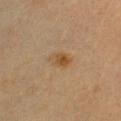workup: imaged on a skin check; not biopsied
acquisition: ~15 mm crop, total-body skin-cancer survey
tile lighting: cross-polarized illumination
patient: female, about 20 years old
image-analysis metrics: a footprint of about 5 mm², an outline eccentricity of about 0.75 (0 = round, 1 = elongated), and a symmetry-axis asymmetry near 0.1; a lesion color around L≈44 a*≈16 b*≈32 in CIELAB, about 7 CIELAB-L* units darker than the surrounding skin, and a lesion-to-skin contrast of about 7 (normalized; higher = more distinct)
body site: the chest
lesion diameter: ~3 mm (longest diameter)